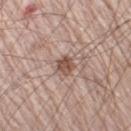acquisition = total-body-photography crop, ~15 mm field of view; subject = male, aged around 65; illumination = white-light illumination; site = the right upper arm; size = ~2.5 mm (longest diameter).